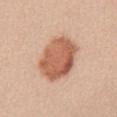Captured during whole-body skin photography for melanoma surveillance; the lesion was not biopsied. A lesion tile, about 15 mm wide, cut from a 3D total-body photograph. Located on the abdomen. Imaged with white-light lighting. The patient is a female approximately 45 years of age. Approximately 5.5 mm at its widest.